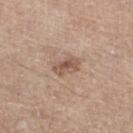biopsy status: imaged on a skin check; not biopsied | location: the left lower leg | imaging modality: 15 mm crop, total-body photography | patient: male, approximately 75 years of age | size: ~3 mm (longest diameter) | tile lighting: white-light.